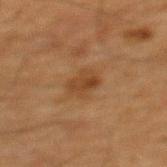tile lighting: cross-polarized | image-analysis metrics: an outline eccentricity of about 0.45 (0 = round, 1 = elongated) and a shape-asymmetry score of about 0.15 (0 = symmetric); a within-lesion color-variation index near 3.5/10 and peripheral color asymmetry of about 1.5; an automated nevus-likeness rating near 25 out of 100 and lesion-presence confidence of about 100/100 | size: ≈3 mm | patient: male, approximately 65 years of age | anatomic site: the mid back | image source: total-body-photography crop, ~15 mm field of view.